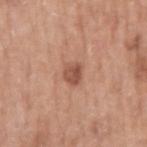No biopsy was performed on this lesion — it was imaged during a full skin examination and was not determined to be concerning. A male patient roughly 65 years of age. An algorithmic analysis of the crop reported an area of roughly 4.5 mm² and a shape-asymmetry score of about 0.2 (0 = symmetric). And it measured a lesion color around L≈52 a*≈23 b*≈29 in CIELAB. And it measured a classifier nevus-likeness of about 60/100 and lesion-presence confidence of about 100/100. About 2.5 mm across. This is a white-light tile. Located on the right upper arm. A 15 mm crop from a total-body photograph taken for skin-cancer surveillance.